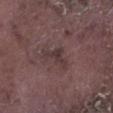biopsy status = catalogued during a skin exam; not biopsied
site = the left lower leg
automated lesion analysis = a lesion area of about 4.5 mm² and a shape eccentricity near 0.85; border irregularity of about 7 on a 0–10 scale and a within-lesion color-variation index near 1/10
illumination = white-light illumination
subject = male, in their mid-70s
acquisition = ~15 mm crop, total-body skin-cancer survey
lesion diameter = ~3.5 mm (longest diameter)